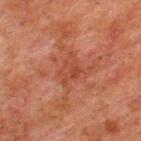workup=total-body-photography surveillance lesion; no biopsy
subject=male, aged around 60
body site=the upper back
image source=total-body-photography crop, ~15 mm field of view